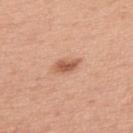Part of a total-body skin-imaging series; this lesion was reviewed on a skin check and was not flagged for biopsy.
A male patient in their 50s.
Captured under white-light illumination.
A roughly 15 mm field-of-view crop from a total-body skin photograph.
Located on the upper back.